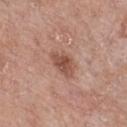biopsy_status: not biopsied; imaged during a skin examination
patient:
  sex: male
  age_approx: 70
image:
  source: total-body photography crop
  field_of_view_mm: 15
lighting: white-light
lesion_size:
  long_diameter_mm_approx: 4.0
site: chest